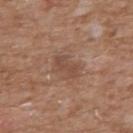Q: Was this lesion biopsied?
A: imaged on a skin check; not biopsied
Q: Who is the patient?
A: male, approximately 60 years of age
Q: Automated lesion metrics?
A: an area of roughly 4 mm², an outline eccentricity of about 0.9 (0 = round, 1 = elongated), and a shape-asymmetry score of about 0.3 (0 = symmetric); a lesion color around L≈47 a*≈20 b*≈27 in CIELAB, a lesion–skin lightness drop of about 7, and a normalized border contrast of about 5.5; lesion-presence confidence of about 100/100
Q: How was this image acquired?
A: total-body-photography crop, ~15 mm field of view
Q: How was the tile lit?
A: white-light illumination
Q: Lesion location?
A: the chest
Q: Lesion size?
A: about 3 mm